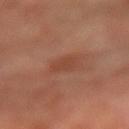Notes:
- workup — no biopsy performed (imaged during a skin exam)
- imaging modality — ~15 mm crop, total-body skin-cancer survey
- site — the left arm
- patient — male, approximately 50 years of age
- illumination — cross-polarized
- lesion diameter — ~2.5 mm (longest diameter)